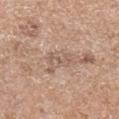The lesion was photographed on a routine skin check and not biopsied; there is no pathology result. A lesion tile, about 15 mm wide, cut from a 3D total-body photograph. The patient is a female aged around 70. The lesion is on the left forearm. This is a white-light tile. The lesion's longest dimension is about 3 mm.